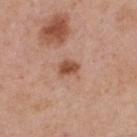  biopsy_status: not biopsied; imaged during a skin examination
  automated_metrics:
    nevus_likeness_0_100: 85
  lesion_size:
    long_diameter_mm_approx: 2.5
  lighting: white-light
  site: upper back
  image:
    source: total-body photography crop
    field_of_view_mm: 15
  patient:
    sex: male
    age_approx: 55